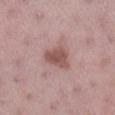workup: no biopsy performed (imaged during a skin exam); image source: total-body-photography crop, ~15 mm field of view; subject: female, approximately 45 years of age; illumination: white-light; location: the right lower leg; size: about 3.5 mm.